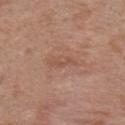Q: Is there a histopathology result?
A: no biopsy performed (imaged during a skin exam)
Q: What kind of image is this?
A: ~15 mm tile from a whole-body skin photo
Q: Lesion size?
A: ~3.5 mm (longest diameter)
Q: What did automated image analysis measure?
A: a footprint of about 3.5 mm², an outline eccentricity of about 0.95 (0 = round, 1 = elongated), and a shape-asymmetry score of about 0.35 (0 = symmetric); a nevus-likeness score of about 0/100 and lesion-presence confidence of about 100/100
Q: How was the tile lit?
A: white-light
Q: Lesion location?
A: the upper back
Q: Patient demographics?
A: female, aged 38–42